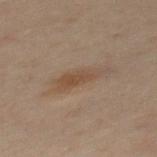The lesion is located on the mid back. A 15 mm close-up tile from a total-body photography series done for melanoma screening. A male subject, aged around 50. Measured at roughly 5 mm in maximum diameter.